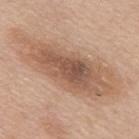notes: imaged on a skin check; not biopsied | location: the back | lesion size: about 13 mm | subject: male, approximately 50 years of age | imaging modality: ~15 mm crop, total-body skin-cancer survey | illumination: white-light.